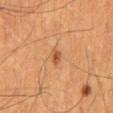| key | value |
|---|---|
| biopsy status | catalogued during a skin exam; not biopsied |
| lighting | cross-polarized |
| automated lesion analysis | a mean CIELAB color near L≈50 a*≈23 b*≈36, a lesion–skin lightness drop of about 9, and a normalized lesion–skin contrast near 6.5; a border-irregularity index near 2.5/10 and peripheral color asymmetry of about 1.5 |
| lesion size | about 2 mm |
| subject | male, aged approximately 60 |
| anatomic site | the back |
| imaging modality | ~15 mm tile from a whole-body skin photo |